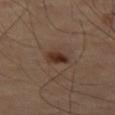Impression:
Part of a total-body skin-imaging series; this lesion was reviewed on a skin check and was not flagged for biopsy.
Context:
The tile uses cross-polarized illumination. The lesion is on the left thigh. A 15 mm close-up tile from a total-body photography series done for melanoma screening.